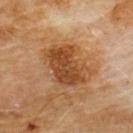Part of a total-body skin-imaging series; this lesion was reviewed on a skin check and was not flagged for biopsy. Cropped from a whole-body photographic skin survey; the tile spans about 15 mm. About 6 mm across. On the chest. A male subject in their 60s. Captured under cross-polarized illumination.